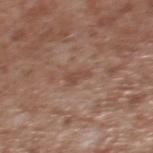Findings:
- notes · total-body-photography surveillance lesion; no biopsy
- size · ≈3 mm
- anatomic site · the upper back
- automated lesion analysis · an eccentricity of roughly 0.85; a mean CIELAB color near L≈47 a*≈19 b*≈26 and a normalized border contrast of about 6; internal color variation of about 0.5 on a 0–10 scale
- tile lighting · white-light
- acquisition · 15 mm crop, total-body photography
- patient · male, aged 43–47